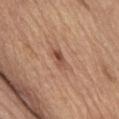Imaged during a routine full-body skin examination; the lesion was not biopsied and no histopathology is available. The patient is a male aged 68 to 72. The total-body-photography lesion software estimated a footprint of about 3.5 mm², an outline eccentricity of about 0.85 (0 = round, 1 = elongated), and two-axis asymmetry of about 0.2. It also reported roughly 10 lightness units darker than nearby skin and a lesion-to-skin contrast of about 7.5 (normalized; higher = more distinct). Measured at roughly 3 mm in maximum diameter. A 15 mm close-up tile from a total-body photography series done for melanoma screening. This is a white-light tile. Located on the front of the torso.